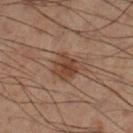biopsy status: no biopsy performed (imaged during a skin exam) | size: about 3.5 mm | automated metrics: a border-irregularity rating of about 2.5/10, a within-lesion color-variation index near 3/10, and peripheral color asymmetry of about 1 | lighting: cross-polarized | site: the right lower leg | imaging modality: ~15 mm tile from a whole-body skin photo | patient: male, aged 48–52.